Measured at roughly 12 mm in maximum diameter; the lesion is located on the left thigh; the subject is a female in their mid-60s; a roughly 15 mm field-of-view crop from a total-body skin photograph; The lesion-visualizer software estimated a border-irregularity rating of about 3/10, a color-variation rating of about 7/10, and peripheral color asymmetry of about 2.5 — 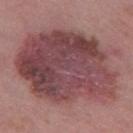Q: What did pathology find?
A: an invasive melanoma, superficial spreading type, Breslow thickness 0.6 mm and mitotic rate <1/mm²